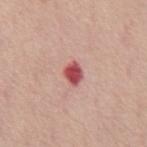Clinical impression: Imaged during a routine full-body skin examination; the lesion was not biopsied and no histopathology is available. Image and clinical context: A male patient, aged approximately 55. The lesion is on the front of the torso. A region of skin cropped from a whole-body photographic capture, roughly 15 mm wide.